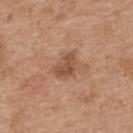Notes:
* notes — catalogued during a skin exam; not biopsied
* tile lighting — white-light
* lesion size — ~3 mm (longest diameter)
* site — the back
* subject — female, aged approximately 40
* imaging modality — ~15 mm tile from a whole-body skin photo